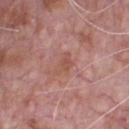Assessment:
Part of a total-body skin-imaging series; this lesion was reviewed on a skin check and was not flagged for biopsy.
Background:
A male patient about 70 years old. The lesion is on the chest. Cropped from a whole-body photographic skin survey; the tile spans about 15 mm.